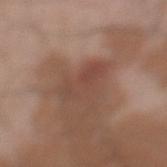{"biopsy_status": "not biopsied; imaged during a skin examination", "patient": {"sex": "male", "age_approx": 25}, "site": "left lower leg", "image": {"source": "total-body photography crop", "field_of_view_mm": 15}}